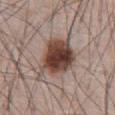Q: Is there a histopathology result?
A: catalogued during a skin exam; not biopsied
Q: What did automated image analysis measure?
A: an average lesion color of about L≈43 a*≈18 b*≈23 (CIELAB) and a normalized lesion–skin contrast near 13
Q: What lighting was used for the tile?
A: white-light illumination
Q: How was this image acquired?
A: ~15 mm tile from a whole-body skin photo
Q: Patient demographics?
A: male, aged around 65
Q: What is the anatomic site?
A: the abdomen